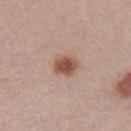Findings:
* notes: catalogued during a skin exam; not biopsied
* acquisition: 15 mm crop, total-body photography
* patient: male, aged approximately 30